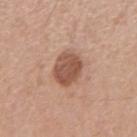Q: Was a biopsy performed?
A: total-body-photography surveillance lesion; no biopsy
Q: How large is the lesion?
A: ~3.5 mm (longest diameter)
Q: Lesion location?
A: the left forearm
Q: Patient demographics?
A: female, roughly 35 years of age
Q: What lighting was used for the tile?
A: white-light illumination
Q: What did automated image analysis measure?
A: a classifier nevus-likeness of about 25/100
Q: How was this image acquired?
A: ~15 mm crop, total-body skin-cancer survey